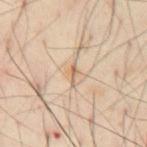Clinical impression: The lesion was tiled from a total-body skin photograph and was not biopsied. Background: The tile uses cross-polarized illumination. Located on the abdomen. A male subject roughly 55 years of age. Cropped from a whole-body photographic skin survey; the tile spans about 15 mm. About 2.5 mm across. The total-body-photography lesion software estimated a mean CIELAB color near L≈65 a*≈16 b*≈33, a lesion–skin lightness drop of about 10, and a lesion-to-skin contrast of about 6.5 (normalized; higher = more distinct). It also reported a border-irregularity rating of about 5.5/10, a within-lesion color-variation index near 0/10, and a peripheral color-asymmetry measure near 0.